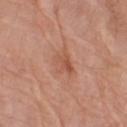biopsy status: catalogued during a skin exam; not biopsied
site: the left forearm
image source: total-body-photography crop, ~15 mm field of view
patient: male, aged approximately 80